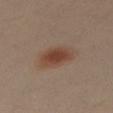- notes — catalogued during a skin exam; not biopsied
- lesion size — ~4 mm (longest diameter)
- acquisition — 15 mm crop, total-body photography
- automated lesion analysis — a mean CIELAB color near L≈43 a*≈20 b*≈27 and roughly 10 lightness units darker than nearby skin; a border-irregularity rating of about 2/10, a within-lesion color-variation index near 3.5/10, and radial color variation of about 1
- site — the leg
- patient — female, aged 33 to 37